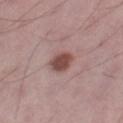Captured during whole-body skin photography for melanoma surveillance; the lesion was not biopsied.
A 15 mm crop from a total-body photograph taken for skin-cancer surveillance.
From the right thigh.
A male patient aged 48–52.